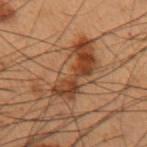workup — total-body-photography surveillance lesion; no biopsy | subject — male, about 55 years old | automated lesion analysis — an area of roughly 19 mm², a shape eccentricity near 0.9, and a symmetry-axis asymmetry near 0.6; a lesion color around L≈35 a*≈19 b*≈28 in CIELAB, roughly 10 lightness units darker than nearby skin, and a normalized lesion–skin contrast near 8.5; border irregularity of about 9 on a 0–10 scale and a peripheral color-asymmetry measure near 2; an automated nevus-likeness rating near 75 out of 100 and a lesion-detection confidence of about 100/100 | image source — total-body-photography crop, ~15 mm field of view | lesion size — about 8.5 mm | anatomic site — the left upper arm | illumination — cross-polarized.